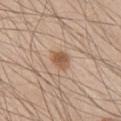No biopsy was performed on this lesion — it was imaged during a full skin examination and was not determined to be concerning. The lesion is located on the chest. This is a white-light tile. Cropped from a total-body skin-imaging series; the visible field is about 15 mm. Measured at roughly 3 mm in maximum diameter. A male subject, roughly 45 years of age.